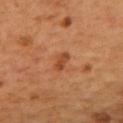notes: imaged on a skin check; not biopsied
subject: male, aged 53–57
acquisition: ~15 mm crop, total-body skin-cancer survey
body site: the mid back
lesion diameter: ~2.5 mm (longest diameter)